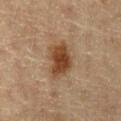Clinical impression:
The lesion was tiled from a total-body skin photograph and was not biopsied.
Context:
A 15 mm close-up extracted from a 3D total-body photography capture. A male patient, about 60 years old.